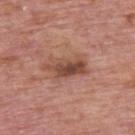image source: ~15 mm tile from a whole-body skin photo | location: the upper back | subject: male, roughly 75 years of age | illumination: white-light | diameter: ≈5 mm.